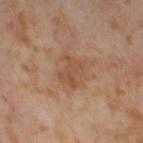Clinical impression:
Captured during whole-body skin photography for melanoma surveillance; the lesion was not biopsied.
Image and clinical context:
Measured at roughly 3.5 mm in maximum diameter. The total-body-photography lesion software estimated a footprint of about 5.5 mm², a shape eccentricity near 0.75, and a shape-asymmetry score of about 0.25 (0 = symmetric). The analysis additionally found a mean CIELAB color near L≈51 a*≈21 b*≈32, a lesion–skin lightness drop of about 7, and a normalized lesion–skin contrast near 6. The software also gave an automated nevus-likeness rating near 0 out of 100 and a detector confidence of about 100 out of 100 that the crop contains a lesion. A female subject, aged 53–57. From the right thigh. Captured under cross-polarized illumination. Cropped from a total-body skin-imaging series; the visible field is about 15 mm.